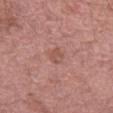Part of a total-body skin-imaging series; this lesion was reviewed on a skin check and was not flagged for biopsy.